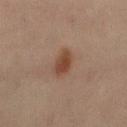Q: Is there a histopathology result?
A: no biopsy performed (imaged during a skin exam)
Q: Where on the body is the lesion?
A: the leg
Q: What is the imaging modality?
A: ~15 mm tile from a whole-body skin photo
Q: Who is the patient?
A: female, roughly 45 years of age